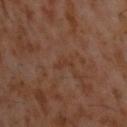Captured during whole-body skin photography for melanoma surveillance; the lesion was not biopsied.
The tile uses cross-polarized illumination.
Cropped from a whole-body photographic skin survey; the tile spans about 15 mm.
The patient is a male in their 60s.
The lesion is on the back.
The recorded lesion diameter is about 2.5 mm.